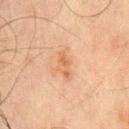workup: catalogued during a skin exam; not biopsied | image: ~15 mm tile from a whole-body skin photo | anatomic site: the chest | patient: male, aged around 70.